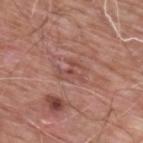The lesion was tiled from a total-body skin photograph and was not biopsied. An algorithmic analysis of the crop reported a lesion color around L≈49 a*≈24 b*≈25 in CIELAB and a lesion–skin lightness drop of about 7. The software also gave a border-irregularity index near 10/10, a color-variation rating of about 0/10, and a peripheral color-asymmetry measure near 0. And it measured an automated nevus-likeness rating near 0 out of 100 and lesion-presence confidence of about 75/100. A male subject, in their 60s. Cropped from a total-body skin-imaging series; the visible field is about 15 mm. The tile uses white-light illumination. The lesion is located on the upper back.